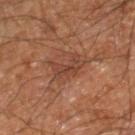<lesion>
  <biopsy_status>not biopsied; imaged during a skin examination</biopsy_status>
  <image>
    <source>total-body photography crop</source>
    <field_of_view_mm>15</field_of_view_mm>
  </image>
  <site>right lower leg</site>
  <patient>
    <sex>male</sex>
    <age_approx>60</age_approx>
  </patient>
</lesion>